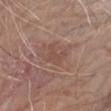tile lighting = white-light illumination; acquisition = 15 mm crop, total-body photography; subject = male, aged 73–77; lesion diameter = ~3.5 mm (longest diameter); body site = the right forearm.